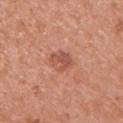The lesion is on the right upper arm. This is a white-light tile. A 15 mm close-up tile from a total-body photography series done for melanoma screening. The lesion's longest dimension is about 3.5 mm. A female patient, about 40 years old.